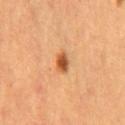| field | value |
|---|---|
| follow-up | total-body-photography surveillance lesion; no biopsy |
| location | the abdomen |
| illumination | cross-polarized |
| patient | male, in their mid-60s |
| image source | ~15 mm tile from a whole-body skin photo |